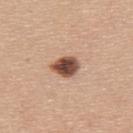Q: Is there a histopathology result?
A: total-body-photography surveillance lesion; no biopsy
Q: What kind of image is this?
A: ~15 mm tile from a whole-body skin photo
Q: How was the tile lit?
A: white-light illumination
Q: What is the lesion's diameter?
A: ≈3.5 mm
Q: Where on the body is the lesion?
A: the upper back
Q: What are the patient's age and sex?
A: female, aged around 40